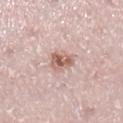biopsy_status: not biopsied; imaged during a skin examination
lesion_size:
  long_diameter_mm_approx: 3.0
image:
  source: total-body photography crop
  field_of_view_mm: 15
site: right lower leg
lighting: white-light
automated_metrics:
  cielab_L: 60
  cielab_a: 20
  cielab_b: 25
  vs_skin_darker_L: 13.0
  border_irregularity_0_10: 3.5
  peripheral_color_asymmetry: 2.5
  nevus_likeness_0_100: 90
  lesion_detection_confidence_0_100: 100
patient:
  sex: female
  age_approx: 25